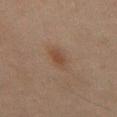On the mid back.
A close-up tile cropped from a whole-body skin photograph, about 15 mm across.
The patient is a male aged around 45.